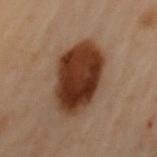  patient:
    sex: female
    age_approx: 60
  image:
    source: total-body photography crop
    field_of_view_mm: 15
  automated_metrics:
    area_mm2_approx: 28.0
    shape_asymmetry: 0.15
    cielab_L: 29
    cielab_a: 19
    cielab_b: 26
    vs_skin_darker_L: 16.0
    vs_skin_contrast_norm: 14.5
    border_irregularity_0_10: 2.0
    color_variation_0_10: 5.0
    peripheral_color_asymmetry: 1.5
    nevus_likeness_0_100: 100
    lesion_detection_confidence_0_100: 100
  site: right arm
  lesion_size:
    long_diameter_mm_approx: 8.0